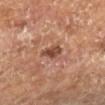- workup: imaged on a skin check; not biopsied
- image source: ~15 mm tile from a whole-body skin photo
- diameter: ≈3 mm
- lighting: cross-polarized illumination
- patient: male, in their mid-60s